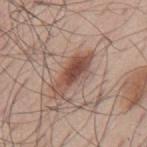<tbp_lesion>
  <biopsy_status>not biopsied; imaged during a skin examination</biopsy_status>
  <site>mid back</site>
  <patient>
    <sex>male</sex>
    <age_approx>55</age_approx>
  </patient>
  <image>
    <source>total-body photography crop</source>
    <field_of_view_mm>15</field_of_view_mm>
  </image>
</tbp_lesion>